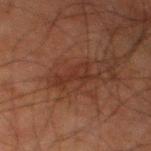Q: Was this lesion biopsied?
A: no biopsy performed (imaged during a skin exam)
Q: How was the tile lit?
A: cross-polarized
Q: How large is the lesion?
A: about 4 mm
Q: How was this image acquired?
A: ~15 mm crop, total-body skin-cancer survey
Q: What are the patient's age and sex?
A: male, roughly 80 years of age
Q: Lesion location?
A: the left thigh